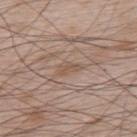  biopsy_status: not biopsied; imaged during a skin examination
  site: back
  image:
    source: total-body photography crop
    field_of_view_mm: 15
  patient:
    sex: male
    age_approx: 65
  automated_metrics:
    area_mm2_approx: 2.5
    eccentricity: 0.9
    border_irregularity_0_10: 3.5
    color_variation_0_10: 0.0
    peripheral_color_asymmetry: 0.0